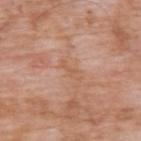  biopsy_status: not biopsied; imaged during a skin examination
  lesion_size:
    long_diameter_mm_approx: 3.0
  image:
    source: total-body photography crop
    field_of_view_mm: 15
  site: back
  patient:
    sex: male
    age_approx: 60